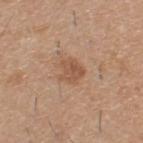| feature | finding |
|---|---|
| notes | catalogued during a skin exam; not biopsied |
| lesion size | ~3 mm (longest diameter) |
| acquisition | 15 mm crop, total-body photography |
| illumination | white-light |
| patient | male, roughly 60 years of age |
| body site | the upper back |